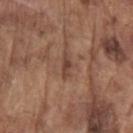Imaged during a routine full-body skin examination; the lesion was not biopsied and no histopathology is available. A region of skin cropped from a whole-body photographic capture, roughly 15 mm wide. A male patient, aged around 80. Measured at roughly 3 mm in maximum diameter. Imaged with white-light lighting. The lesion is located on the left upper arm.